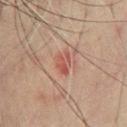Imaged during a routine full-body skin examination; the lesion was not biopsied and no histopathology is available.
Measured at roughly 3 mm in maximum diameter.
The patient is a male in their 60s.
On the chest.
Imaged with cross-polarized lighting.
A 15 mm close-up tile from a total-body photography series done for melanoma screening.